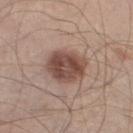• notes — imaged on a skin check; not biopsied
• tile lighting — white-light
• site — the left thigh
• image — ~15 mm tile from a whole-body skin photo
• subject — male, about 55 years old
• automated lesion analysis — an area of roughly 14 mm², an outline eccentricity of about 0.5 (0 = round, 1 = elongated), and two-axis asymmetry of about 0.15; a mean CIELAB color near L≈47 a*≈19 b*≈24, about 14 CIELAB-L* units darker than the surrounding skin, and a normalized border contrast of about 10